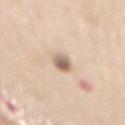| key | value |
|---|---|
| workup | catalogued during a skin exam; not biopsied |
| acquisition | ~15 mm tile from a whole-body skin photo |
| patient | female, approximately 65 years of age |
| site | the mid back |
| tile lighting | white-light |